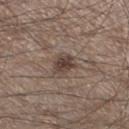Case summary:
• workup — catalogued during a skin exam; not biopsied
• imaging modality — 15 mm crop, total-body photography
• site — the left lower leg
• patient — male, roughly 45 years of age
• automated metrics — an area of roughly 6 mm², an outline eccentricity of about 0.65 (0 = round, 1 = elongated), and a shape-asymmetry score of about 0.25 (0 = symmetric)
• tile lighting — white-light illumination
• size — ≈3 mm